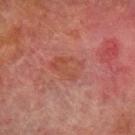<record>
<patient>
  <sex>male</sex>
  <age_approx>75</age_approx>
</patient>
<lesion_size>
  <long_diameter_mm_approx>4.0</long_diameter_mm_approx>
</lesion_size>
<site>left forearm</site>
<image>
  <source>total-body photography crop</source>
  <field_of_view_mm>15</field_of_view_mm>
</image>
<lighting>cross-polarized</lighting>
</record>